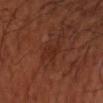{"biopsy_status": "not biopsied; imaged during a skin examination", "image": {"source": "total-body photography crop", "field_of_view_mm": 15}, "lesion_size": {"long_diameter_mm_approx": 3.0}, "patient": {"sex": "male", "age_approx": 50}, "lighting": "cross-polarized", "site": "right forearm"}